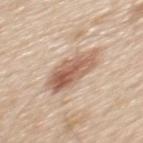| feature | finding |
|---|---|
| workup | no biopsy performed (imaged during a skin exam) |